A female patient roughly 70 years of age. A roughly 15 mm field-of-view crop from a total-body skin photograph. Located on the right lower leg: 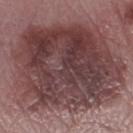diameter: about 13 mm | automated metrics: a lesion area of about 100 mm², an outline eccentricity of about 0.65 (0 = round, 1 = elongated), and two-axis asymmetry of about 0.15; about 13 CIELAB-L* units darker than the surrounding skin and a lesion-to-skin contrast of about 10 (normalized; higher = more distinct); a border-irregularity rating of about 3/10, a within-lesion color-variation index near 8/10, and peripheral color asymmetry of about 3 | biopsy diagnosis: an invasive melanoma, superficial spreading type; Breslow depth 0.3 mm, mitotic rate <1/mm².